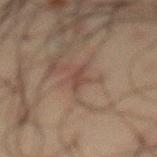Q: Is there a histopathology result?
A: catalogued during a skin exam; not biopsied
Q: Illumination type?
A: cross-polarized
Q: How was this image acquired?
A: 15 mm crop, total-body photography
Q: What did automated image analysis measure?
A: an automated nevus-likeness rating near 0 out of 100 and lesion-presence confidence of about 75/100
Q: Where on the body is the lesion?
A: the front of the torso
Q: What is the lesion's diameter?
A: about 2.5 mm
Q: What are the patient's age and sex?
A: male, aged around 60